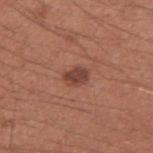{
  "biopsy_status": "not biopsied; imaged during a skin examination",
  "patient": {
    "sex": "male",
    "age_approx": 35
  },
  "site": "arm",
  "automated_metrics": {
    "cielab_L": 43,
    "cielab_a": 23,
    "cielab_b": 26,
    "vs_skin_darker_L": 10.0,
    "vs_skin_contrast_norm": 8.0,
    "border_irregularity_0_10": 2.0,
    "color_variation_0_10": 2.5,
    "peripheral_color_asymmetry": 1.0,
    "nevus_likeness_0_100": 70,
    "lesion_detection_confidence_0_100": 100
  },
  "image": {
    "source": "total-body photography crop",
    "field_of_view_mm": 15
  },
  "lesion_size": {
    "long_diameter_mm_approx": 2.5
  }
}